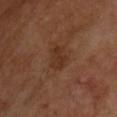| feature | finding |
|---|---|
| lesion diameter | ≈3 mm |
| lighting | cross-polarized illumination |
| TBP lesion metrics | an outline eccentricity of about 0.7 (0 = round, 1 = elongated) and a shape-asymmetry score of about 0.35 (0 = symmetric); a border-irregularity rating of about 3.5/10 and a peripheral color-asymmetry measure near 0.5; a classifier nevus-likeness of about 0/100 and lesion-presence confidence of about 100/100 |
| patient | male, aged 63 to 67 |
| imaging modality | 15 mm crop, total-body photography |